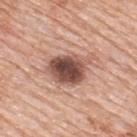  patient:
    sex: male
    age_approx: 80
  automated_metrics:
    cielab_L: 52
    cielab_a: 21
    cielab_b: 25
    vs_skin_darker_L: 17.0
    vs_skin_contrast_norm: 11.5
    nevus_likeness_0_100: 95
    lesion_detection_confidence_0_100: 100
  site: upper back
  image:
    source: total-body photography crop
    field_of_view_mm: 15